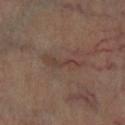Q: Was this lesion biopsied?
A: catalogued during a skin exam; not biopsied
Q: How was the tile lit?
A: cross-polarized
Q: Where on the body is the lesion?
A: the left lower leg
Q: What are the patient's age and sex?
A: aged 63–67
Q: Automated lesion metrics?
A: an area of roughly 5 mm², an outline eccentricity of about 0.95 (0 = round, 1 = elongated), and two-axis asymmetry of about 0.5; a lesion color around L≈38 a*≈16 b*≈22 in CIELAB and a lesion–skin lightness drop of about 6
Q: What kind of image is this?
A: 15 mm crop, total-body photography